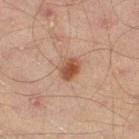The lesion was photographed on a routine skin check and not biopsied; there is no pathology result. The lesion's longest dimension is about 3 mm. The total-body-photography lesion software estimated a footprint of about 5 mm², an outline eccentricity of about 0.7 (0 = round, 1 = elongated), and a shape-asymmetry score of about 0.15 (0 = symmetric). The analysis additionally found a mean CIELAB color near L≈41 a*≈20 b*≈27, about 10 CIELAB-L* units darker than the surrounding skin, and a lesion-to-skin contrast of about 9 (normalized; higher = more distinct). A male subject, in their mid- to late 40s. The lesion is on the left thigh. A lesion tile, about 15 mm wide, cut from a 3D total-body photograph.